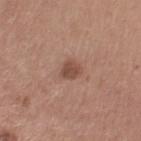Findings:
• image: ~15 mm tile from a whole-body skin photo
• body site: the arm
• diameter: ~2.5 mm (longest diameter)
• lighting: white-light illumination
• image-analysis metrics: a border-irregularity rating of about 1.5/10, a color-variation rating of about 2/10, and a peripheral color-asymmetry measure near 1
• subject: female, approximately 30 years of age